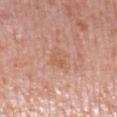<lesion>
<biopsy_status>not biopsied; imaged during a skin examination</biopsy_status>
<site>right lower leg</site>
<lighting>white-light</lighting>
<image>
  <source>total-body photography crop</source>
  <field_of_view_mm>15</field_of_view_mm>
</image>
<patient>
  <sex>female</sex>
  <age_approx>40</age_approx>
</patient>
<automated_metrics>
  <border_irregularity_0_10>2.0</border_irregularity_0_10>
  <color_variation_0_10>3.0</color_variation_0_10>
  <peripheral_color_asymmetry>1.0</peripheral_color_asymmetry>
  <nevus_likeness_0_100>0</nevus_likeness_0_100>
</automated_metrics>
</lesion>